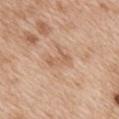The lesion was photographed on a routine skin check and not biopsied; there is no pathology result. The patient is a female roughly 40 years of age. A lesion tile, about 15 mm wide, cut from a 3D total-body photograph. On the mid back.